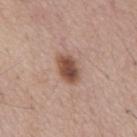notes: catalogued during a skin exam; not biopsied | tile lighting: white-light illumination | image: 15 mm crop, total-body photography | subject: male, about 55 years old | automated lesion analysis: a border-irregularity index near 1.5/10 and a color-variation rating of about 5/10; a classifier nevus-likeness of about 100/100 and a lesion-detection confidence of about 100/100 | size: ~3.5 mm (longest diameter).